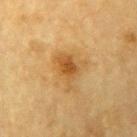No biopsy was performed on this lesion — it was imaged during a full skin examination and was not determined to be concerning.
Longest diameter approximately 4.5 mm.
From the left upper arm.
The subject is a male about 85 years old or older.
A region of skin cropped from a whole-body photographic capture, roughly 15 mm wide.
Imaged with cross-polarized lighting.